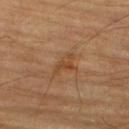This lesion was catalogued during total-body skin photography and was not selected for biopsy. Imaged with cross-polarized lighting. A 15 mm close-up extracted from a 3D total-body photography capture. The lesion is on the right thigh. A male patient, roughly 85 years of age or older.